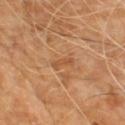Imaged during a routine full-body skin examination; the lesion was not biopsied and no histopathology is available. A male patient roughly 70 years of age. A 15 mm close-up extracted from a 3D total-body photography capture. The recorded lesion diameter is about 3 mm. The lesion is located on the front of the torso.